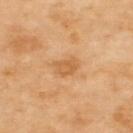Clinical impression: Captured during whole-body skin photography for melanoma surveillance; the lesion was not biopsied. Image and clinical context: This image is a 15 mm lesion crop taken from a total-body photograph. On the upper back. Captured under cross-polarized illumination. About 3 mm across. An algorithmic analysis of the crop reported a lesion color around L≈62 a*≈23 b*≈43 in CIELAB, about 8 CIELAB-L* units darker than the surrounding skin, and a normalized border contrast of about 6. It also reported a border-irregularity rating of about 3/10 and peripheral color asymmetry of about 0.5.